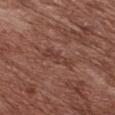This lesion was catalogued during total-body skin photography and was not selected for biopsy. About 4.5 mm across. A female patient, approximately 80 years of age. Captured under white-light illumination. A 15 mm close-up extracted from a 3D total-body photography capture. Automated tile analysis of the lesion measured a footprint of about 5.5 mm², an outline eccentricity of about 0.95 (0 = round, 1 = elongated), and two-axis asymmetry of about 0.3. The software also gave a border-irregularity rating of about 4/10 and internal color variation of about 1.5 on a 0–10 scale. The software also gave an automated nevus-likeness rating near 0 out of 100 and a lesion-detection confidence of about 70/100. The lesion is located on the chest.